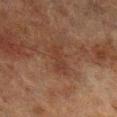{
  "biopsy_status": "not biopsied; imaged during a skin examination",
  "site": "right lower leg",
  "lighting": "cross-polarized",
  "lesion_size": {
    "long_diameter_mm_approx": 4.0
  },
  "automated_metrics": {
    "area_mm2_approx": 5.0,
    "eccentricity": 0.9,
    "shape_asymmetry": 0.4,
    "cielab_L": 31,
    "cielab_a": 18,
    "cielab_b": 24,
    "vs_skin_darker_L": 5.0,
    "vs_skin_contrast_norm": 5.0
  },
  "image": {
    "source": "total-body photography crop",
    "field_of_view_mm": 15
  },
  "patient": {
    "sex": "male",
    "age_approx": 70
  }
}